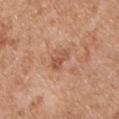Impression: The lesion was tiled from a total-body skin photograph and was not biopsied. Clinical summary: A male patient, aged 63 to 67. The lesion is located on the chest. Measured at roughly 3 mm in maximum diameter. This image is a 15 mm lesion crop taken from a total-body photograph.